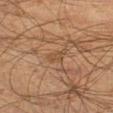On the right thigh.
A roughly 15 mm field-of-view crop from a total-body skin photograph.
Measured at roughly 2.5 mm in maximum diameter.
The total-body-photography lesion software estimated a mean CIELAB color near L≈40 a*≈15 b*≈29. It also reported border irregularity of about 3.5 on a 0–10 scale, a within-lesion color-variation index near 1/10, and peripheral color asymmetry of about 0.5.
A male subject, aged 58 to 62.
Captured under cross-polarized illumination.